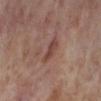Findings:
• notes: imaged on a skin check; not biopsied
• diameter: ~3.5 mm (longest diameter)
• illumination: cross-polarized
• image: ~15 mm crop, total-body skin-cancer survey
• subject: female, aged approximately 55
• automated lesion analysis: a shape eccentricity near 0.95; a lesion color around L≈44 a*≈21 b*≈25 in CIELAB
• location: the right lower leg